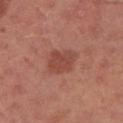{"biopsy_status": "not biopsied; imaged during a skin examination", "image": {"source": "total-body photography crop", "field_of_view_mm": 15}, "lesion_size": {"long_diameter_mm_approx": 3.5}, "lighting": "white-light", "site": "left upper arm", "automated_metrics": {"area_mm2_approx": 8.0, "eccentricity": 0.6, "cielab_L": 46, "cielab_a": 27, "cielab_b": 28, "vs_skin_darker_L": 8.0, "vs_skin_contrast_norm": 6.5, "border_irregularity_0_10": 2.5, "color_variation_0_10": 2.5, "peripheral_color_asymmetry": 1.0}, "patient": {"sex": "male", "age_approx": 30}}